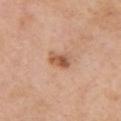Part of a total-body skin-imaging series; this lesion was reviewed on a skin check and was not flagged for biopsy. The tile uses white-light illumination. Measured at roughly 3 mm in maximum diameter. A region of skin cropped from a whole-body photographic capture, roughly 15 mm wide. Located on the chest. The subject is a female aged around 55.